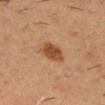Assessment: No biopsy was performed on this lesion — it was imaged during a full skin examination and was not determined to be concerning. Background: This image is a 15 mm lesion crop taken from a total-body photograph. About 3.5 mm across. A male subject, aged 33–37. Located on the head or neck. The tile uses cross-polarized illumination.